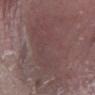Acquisition and patient details: Automated tile analysis of the lesion measured an average lesion color of about L≈43 a*≈17 b*≈17 (CIELAB), a lesion–skin lightness drop of about 5, and a normalized border contrast of about 4.5. And it measured border irregularity of about 8 on a 0–10 scale, a color-variation rating of about 3.5/10, and a peripheral color-asymmetry measure near 1.5. From the right lower leg. This is a white-light tile. About 9 mm across. A male subject in their 80s. A 15 mm close-up extracted from a 3D total-body photography capture.